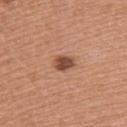Part of a total-body skin-imaging series; this lesion was reviewed on a skin check and was not flagged for biopsy. The lesion-visualizer software estimated an average lesion color of about L≈48 a*≈24 b*≈30 (CIELAB) and a lesion–skin lightness drop of about 14. The software also gave a border-irregularity rating of about 1.5/10. Cropped from a whole-body photographic skin survey; the tile spans about 15 mm. A female patient, approximately 40 years of age. From the back. Imaged with white-light lighting.